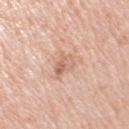{"biopsy_status": "not biopsied; imaged during a skin examination", "image": {"source": "total-body photography crop", "field_of_view_mm": 15}, "patient": {"sex": "female", "age_approx": 70}, "site": "right upper arm"}